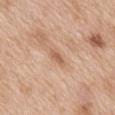Impression: The lesion was tiled from a total-body skin photograph and was not biopsied. Background: About 2.5 mm across. A close-up tile cropped from a whole-body skin photograph, about 15 mm across. Captured under white-light illumination. From the mid back. A female subject aged 38 to 42.